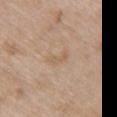  biopsy_status: not biopsied; imaged during a skin examination
  image:
    source: total-body photography crop
    field_of_view_mm: 15
  site: upper back
  patient:
    sex: female
    age_approx: 60